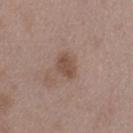biopsy_status: not biopsied; imaged during a skin examination
lighting: white-light
automated_metrics:
  area_mm2_approx: 5.5
  eccentricity: 0.65
  cielab_L: 49
  cielab_a: 17
  cielab_b: 25
  vs_skin_contrast_norm: 7.0
  nevus_likeness_0_100: 10
  lesion_detection_confidence_0_100: 100
image:
  source: total-body photography crop
  field_of_view_mm: 15
lesion_size:
  long_diameter_mm_approx: 3.0
patient:
  sex: male
  age_approx: 50
site: left thigh